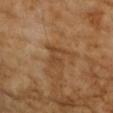Imaged during a routine full-body skin examination; the lesion was not biopsied and no histopathology is available. The patient is a female aged approximately 70. Approximately 3 mm at its widest. This is a cross-polarized tile. This image is a 15 mm lesion crop taken from a total-body photograph. The lesion is on the right upper arm.